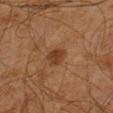<case>
  <biopsy_status>not biopsied; imaged during a skin examination</biopsy_status>
  <image>
    <source>total-body photography crop</source>
    <field_of_view_mm>15</field_of_view_mm>
  </image>
  <patient>
    <sex>male</sex>
    <age_approx>45</age_approx>
  </patient>
  <automated_metrics>
    <nevus_likeness_0_100>55</nevus_likeness_0_100>
    <lesion_detection_confidence_0_100>100</lesion_detection_confidence_0_100>
  </automated_metrics>
  <site>arm</site>
</case>